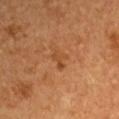Impression:
Captured during whole-body skin photography for melanoma surveillance; the lesion was not biopsied.
Acquisition and patient details:
Longest diameter approximately 2.5 mm. A 15 mm close-up extracted from a 3D total-body photography capture. Captured under cross-polarized illumination. A male subject, aged approximately 55. On the left upper arm.